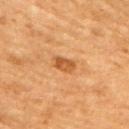Impression:
Recorded during total-body skin imaging; not selected for excision or biopsy.
Context:
Located on the upper back. The recorded lesion diameter is about 3 mm. Automated tile analysis of the lesion measured a classifier nevus-likeness of about 60/100 and a lesion-detection confidence of about 100/100. The subject is a female roughly 55 years of age. This image is a 15 mm lesion crop taken from a total-body photograph.